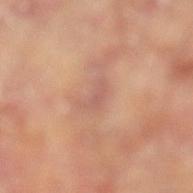workup — catalogued during a skin exam; not biopsied
image source — total-body-photography crop, ~15 mm field of view
patient — female, aged approximately 75
location — the right lower leg